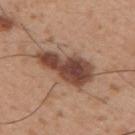biopsy_status: not biopsied; imaged during a skin examination
site: arm
lighting: white-light
patient:
  sex: male
  age_approx: 30
automated_metrics:
  area_mm2_approx: 19.0
  shape_asymmetry: 0.35
  cielab_L: 45
  cielab_a: 20
  cielab_b: 27
  vs_skin_darker_L: 16.0
  vs_skin_contrast_norm: 11.5
lesion_size:
  long_diameter_mm_approx: 7.5
image:
  source: total-body photography crop
  field_of_view_mm: 15